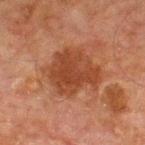Clinical impression:
Imaged during a routine full-body skin examination; the lesion was not biopsied and no histopathology is available.
Context:
Captured under cross-polarized illumination. A 15 mm close-up extracted from a 3D total-body photography capture. The lesion's longest dimension is about 5.5 mm. The lesion is located on the chest. A male patient, roughly 65 years of age.